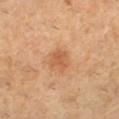Findings:
* workup — no biopsy performed (imaged during a skin exam)
* lesion diameter — ~2.5 mm (longest diameter)
* automated metrics — roughly 9 lightness units darker than nearby skin
* subject — female, aged approximately 30
* imaging modality — ~15 mm tile from a whole-body skin photo
* location — the leg
* illumination — cross-polarized illumination